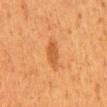Clinical impression:
This lesion was catalogued during total-body skin photography and was not selected for biopsy.
Image and clinical context:
The total-body-photography lesion software estimated a classifier nevus-likeness of about 85/100. The lesion is located on the mid back. A male patient, aged approximately 45. Longest diameter approximately 3.5 mm. The tile uses cross-polarized illumination. A roughly 15 mm field-of-view crop from a total-body skin photograph.